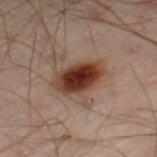Impression: No biopsy was performed on this lesion — it was imaged during a full skin examination and was not determined to be concerning. Clinical summary: The patient is a male approximately 50 years of age. The lesion is located on the left leg. This is a cross-polarized tile. Automated tile analysis of the lesion measured a symmetry-axis asymmetry near 0.15. And it measured border irregularity of about 2 on a 0–10 scale, internal color variation of about 6.5 on a 0–10 scale, and peripheral color asymmetry of about 2. The analysis additionally found an automated nevus-likeness rating near 100 out of 100 and lesion-presence confidence of about 100/100. A 15 mm close-up tile from a total-body photography series done for melanoma screening. Measured at roughly 5 mm in maximum diameter.